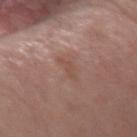follow-up=imaged on a skin check; not biopsied | diameter=≈2.5 mm | patient=male, roughly 70 years of age | image=~15 mm crop, total-body skin-cancer survey | site=the left forearm | tile lighting=white-light.